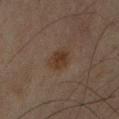notes = imaged on a skin check; not biopsied | location = the right upper arm | subject = male, aged around 65 | image source = total-body-photography crop, ~15 mm field of view.